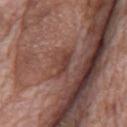Impression: Part of a total-body skin-imaging series; this lesion was reviewed on a skin check and was not flagged for biopsy. Acquisition and patient details: The lesion is located on the mid back. Cropped from a whole-body photographic skin survey; the tile spans about 15 mm. A male patient roughly 70 years of age.